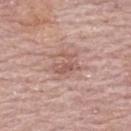biopsy status=catalogued during a skin exam; not biopsied
TBP lesion metrics=a mean CIELAB color near L≈56 a*≈22 b*≈25 and a lesion–skin lightness drop of about 8; border irregularity of about 6.5 on a 0–10 scale, a within-lesion color-variation index near 2/10, and radial color variation of about 0.5; a nevus-likeness score of about 0/100 and a lesion-detection confidence of about 100/100
image source=total-body-photography crop, ~15 mm field of view
anatomic site=the upper back
tile lighting=white-light illumination
subject=female, in their mid-60s